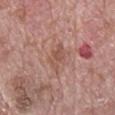Assessment:
Captured during whole-body skin photography for melanoma surveillance; the lesion was not biopsied.
Image and clinical context:
An algorithmic analysis of the crop reported a lesion area of about 5.5 mm² and an outline eccentricity of about 0.9 (0 = round, 1 = elongated). The software also gave an average lesion color of about L≈53 a*≈21 b*≈27 (CIELAB), about 7 CIELAB-L* units darker than the surrounding skin, and a lesion-to-skin contrast of about 6 (normalized; higher = more distinct). The analysis additionally found a border-irregularity rating of about 4.5/10, internal color variation of about 2.5 on a 0–10 scale, and radial color variation of about 1. And it measured a nevus-likeness score of about 0/100 and a detector confidence of about 95 out of 100 that the crop contains a lesion. Located on the mid back. This is a white-light tile. A 15 mm close-up tile from a total-body photography series done for melanoma screening. Longest diameter approximately 4 mm. A male subject aged 68–72.